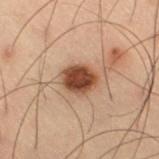Assessment:
Part of a total-body skin-imaging series; this lesion was reviewed on a skin check and was not flagged for biopsy.
Acquisition and patient details:
From the right thigh. Approximately 4 mm at its widest. Captured under cross-polarized illumination. Automated tile analysis of the lesion measured a border-irregularity rating of about 1.5/10, internal color variation of about 3 on a 0–10 scale, and radial color variation of about 1. Cropped from a total-body skin-imaging series; the visible field is about 15 mm. A male subject, aged around 55.